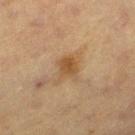{
  "biopsy_status": "not biopsied; imaged during a skin examination",
  "patient": {
    "sex": "female",
    "age_approx": 55
  },
  "lesion_size": {
    "long_diameter_mm_approx": 3.0
  },
  "image": {
    "source": "total-body photography crop",
    "field_of_view_mm": 15
  },
  "lighting": "cross-polarized",
  "automated_metrics": {
    "area_mm2_approx": 5.5,
    "eccentricity": 0.55,
    "border_irregularity_0_10": 3.5,
    "color_variation_0_10": 2.5,
    "peripheral_color_asymmetry": 0.5
  },
  "site": "left thigh"
}